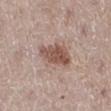{"biopsy_status": "not biopsied; imaged during a skin examination", "automated_metrics": {"area_mm2_approx": 9.5, "eccentricity": 0.85, "shape_asymmetry": 0.25, "cielab_L": 52, "cielab_a": 19, "cielab_b": 24, "vs_skin_darker_L": 13.0, "vs_skin_contrast_norm": 9.0}, "site": "right lower leg", "patient": {"sex": "female", "age_approx": 50}, "lesion_size": {"long_diameter_mm_approx": 5.0}, "image": {"source": "total-body photography crop", "field_of_view_mm": 15}, "lighting": "white-light"}